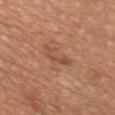{"biopsy_status": "not biopsied; imaged during a skin examination", "site": "chest", "lesion_size": {"long_diameter_mm_approx": 4.5}, "patient": {"sex": "female", "age_approx": 60}, "image": {"source": "total-body photography crop", "field_of_view_mm": 15}, "lighting": "white-light"}